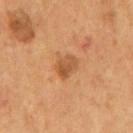No biopsy was performed on this lesion — it was imaged during a full skin examination and was not determined to be concerning.
An algorithmic analysis of the crop reported a footprint of about 5 mm² and a shape eccentricity near 0.7. The analysis additionally found a border-irregularity index near 2/10 and a peripheral color-asymmetry measure near 1.5.
Imaged with cross-polarized lighting.
The lesion is located on the mid back.
Approximately 3 mm at its widest.
A male patient, aged around 55.
A lesion tile, about 15 mm wide, cut from a 3D total-body photograph.